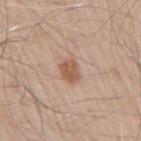Findings:
• follow-up: catalogued during a skin exam; not biopsied
• patient: male, aged 68 to 72
• illumination: white-light illumination
• location: the right upper arm
• image source: 15 mm crop, total-body photography
• TBP lesion metrics: a lesion area of about 5.5 mm² and a symmetry-axis asymmetry near 0.25; a border-irregularity index near 2.5/10, internal color variation of about 2.5 on a 0–10 scale, and radial color variation of about 1; a classifier nevus-likeness of about 65/100 and a lesion-detection confidence of about 100/100